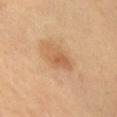The lesion was tiled from a total-body skin photograph and was not biopsied. This is a cross-polarized tile. A male subject about 60 years old. On the right upper arm. Automated image analysis of the tile measured a lesion color around L≈57 a*≈20 b*≈36 in CIELAB, a lesion–skin lightness drop of about 8, and a normalized border contrast of about 6. And it measured a nevus-likeness score of about 40/100 and a lesion-detection confidence of about 100/100. A 15 mm close-up tile from a total-body photography series done for melanoma screening. The lesion's longest dimension is about 4 mm.